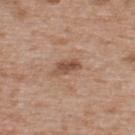| feature | finding |
|---|---|
| biopsy status | no biopsy performed (imaged during a skin exam) |
| tile lighting | white-light |
| diameter | ≈3 mm |
| subject | male, aged 63 to 67 |
| image source | ~15 mm tile from a whole-body skin photo |
| body site | the upper back |